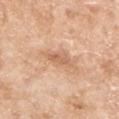Recorded during total-body skin imaging; not selected for excision or biopsy.
The tile uses white-light illumination.
A lesion tile, about 15 mm wide, cut from a 3D total-body photograph.
A female patient approximately 75 years of age.
Located on the arm.